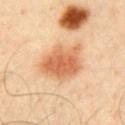Q: Was a biopsy performed?
A: no biopsy performed (imaged during a skin exam)
Q: What is the anatomic site?
A: the chest
Q: Illumination type?
A: cross-polarized
Q: Automated lesion metrics?
A: an average lesion color of about L≈66 a*≈25 b*≈40 (CIELAB), a lesion–skin lightness drop of about 13, and a normalized lesion–skin contrast near 8
Q: How was this image acquired?
A: 15 mm crop, total-body photography
Q: Who is the patient?
A: male, aged around 40
Q: How large is the lesion?
A: about 6 mm